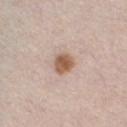biopsy status = catalogued during a skin exam; not biopsied | tile lighting = white-light illumination | image = 15 mm crop, total-body photography | lesion size = ~2.5 mm (longest diameter) | TBP lesion metrics = a lesion area of about 5.5 mm², a shape eccentricity near 0.45, and a symmetry-axis asymmetry near 0.2; a border-irregularity rating of about 2/10 and internal color variation of about 3.5 on a 0–10 scale; an automated nevus-likeness rating near 95 out of 100 and a lesion-detection confidence of about 100/100 | patient = male, in their 30s.